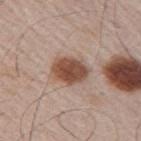Clinical impression: Part of a total-body skin-imaging series; this lesion was reviewed on a skin check and was not flagged for biopsy. Background: This is a white-light tile. A male subject in their mid-60s. A lesion tile, about 15 mm wide, cut from a 3D total-body photograph. On the right upper arm. Measured at roughly 4.5 mm in maximum diameter.